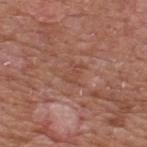Context: A roughly 15 mm field-of-view crop from a total-body skin photograph. Located on the upper back. About 2.5 mm across. A male patient aged around 65.